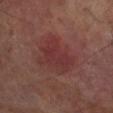| key | value |
|---|---|
| notes | catalogued during a skin exam; not biopsied |
| diameter | ~4.5 mm (longest diameter) |
| patient | male, aged 68–72 |
| image source | 15 mm crop, total-body photography |
| site | the right lower leg |
| TBP lesion metrics | an area of roughly 12 mm², an outline eccentricity of about 0.75 (0 = round, 1 = elongated), and two-axis asymmetry of about 0.3 |